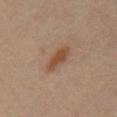follow-up: catalogued during a skin exam; not biopsied
body site: the front of the torso
subject: male, in their 40s
imaging modality: total-body-photography crop, ~15 mm field of view